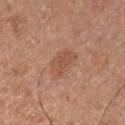No biopsy was performed on this lesion — it was imaged during a full skin examination and was not determined to be concerning. About 3.5 mm across. From the chest. The lesion-visualizer software estimated a footprint of about 6.5 mm² and an outline eccentricity of about 0.8 (0 = round, 1 = elongated). It also reported a border-irregularity rating of about 3.5/10 and a within-lesion color-variation index near 2/10. It also reported an automated nevus-likeness rating near 25 out of 100. This image is a 15 mm lesion crop taken from a total-body photograph. The tile uses white-light illumination. A male subject roughly 30 years of age.